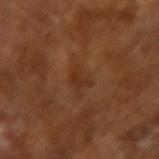Imaged during a routine full-body skin examination; the lesion was not biopsied and no histopathology is available. Automated tile analysis of the lesion measured a footprint of about 2.5 mm², an eccentricity of roughly 0.8, and a shape-asymmetry score of about 0.65 (0 = symmetric). And it measured border irregularity of about 6.5 on a 0–10 scale, internal color variation of about 0 on a 0–10 scale, and peripheral color asymmetry of about 0. Captured under cross-polarized illumination. Cropped from a whole-body photographic skin survey; the tile spans about 15 mm. About 2.5 mm across. A male subject, aged 63 to 67.